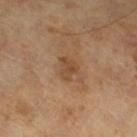follow-up: no biopsy performed (imaged during a skin exam).